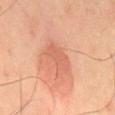biopsy status: no biopsy performed (imaged during a skin exam)
imaging modality: ~15 mm tile from a whole-body skin photo
automated metrics: a footprint of about 7 mm² and an outline eccentricity of about 0.85 (0 = round, 1 = elongated); a mean CIELAB color near L≈62 a*≈28 b*≈34
lesion size: ~4 mm (longest diameter)
body site: the mid back
tile lighting: cross-polarized illumination
patient: male, roughly 65 years of age